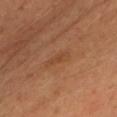The lesion was photographed on a routine skin check and not biopsied; there is no pathology result. About 3 mm across. Located on the chest. The lesion-visualizer software estimated a mean CIELAB color near L≈46 a*≈23 b*≈35, a lesion–skin lightness drop of about 6, and a lesion-to-skin contrast of about 5 (normalized; higher = more distinct). It also reported a border-irregularity rating of about 4/10, a within-lesion color-variation index near 0/10, and radial color variation of about 0. The analysis additionally found an automated nevus-likeness rating near 0 out of 100 and lesion-presence confidence of about 100/100. A female subject, aged approximately 60. A 15 mm crop from a total-body photograph taken for skin-cancer surveillance. The tile uses cross-polarized illumination.